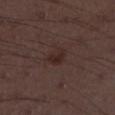Assessment: No biopsy was performed on this lesion — it was imaged during a full skin examination and was not determined to be concerning. Image and clinical context: A lesion tile, about 15 mm wide, cut from a 3D total-body photograph. From the left lower leg. A male patient, aged around 50.